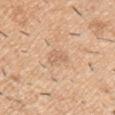Notes:
- workup · total-body-photography surveillance lesion; no biopsy
- image source · ~15 mm tile from a whole-body skin photo
- patient · male, aged 38–42
- site · the left upper arm
- lesion diameter · about 2.5 mm